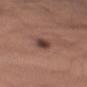Case summary:
– workup: no biopsy performed (imaged during a skin exam)
– tile lighting: white-light illumination
– size: ≈2.5 mm
– image: ~15 mm tile from a whole-body skin photo
– subject: male, aged around 45
– body site: the left upper arm
– TBP lesion metrics: a border-irregularity rating of about 2.5/10 and a within-lesion color-variation index near 4/10; a nevus-likeness score of about 85/100 and lesion-presence confidence of about 100/100